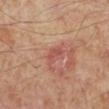Imaged during a routine full-body skin examination; the lesion was not biopsied and no histopathology is available.
An algorithmic analysis of the crop reported border irregularity of about 3.5 on a 0–10 scale, a color-variation rating of about 0.5/10, and a peripheral color-asymmetry measure near 0.
Cropped from a whole-body photographic skin survey; the tile spans about 15 mm.
From the left lower leg.
Captured under cross-polarized illumination.
A male subject aged around 50.
The lesion's longest dimension is about 3 mm.